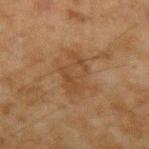<tbp_lesion>
<biopsy_status>not biopsied; imaged during a skin examination</biopsy_status>
<patient>
  <sex>male</sex>
  <age_approx>45</age_approx>
</patient>
<image>
  <source>total-body photography crop</source>
  <field_of_view_mm>15</field_of_view_mm>
</image>
<automated_metrics>
  <cielab_L>37</cielab_L>
  <cielab_a>16</cielab_a>
  <cielab_b>29</cielab_b>
  <vs_skin_darker_L>5.0</vs_skin_darker_L>
  <vs_skin_contrast_norm>5.0</vs_skin_contrast_norm>
  <peripheral_color_asymmetry>1.0</peripheral_color_asymmetry>
</automated_metrics>
<site>left upper arm</site>
</tbp_lesion>